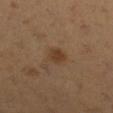The lesion was tiled from a total-body skin photograph and was not biopsied. The patient is a female in their mid-50s. On the right lower leg. A roughly 15 mm field-of-view crop from a total-body skin photograph.